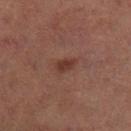{"biopsy_status": "not biopsied; imaged during a skin examination", "site": "right thigh", "patient": {"sex": "female", "age_approx": 60}, "image": {"source": "total-body photography crop", "field_of_view_mm": 15}}